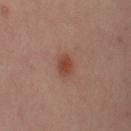Part of a total-body skin-imaging series; this lesion was reviewed on a skin check and was not flagged for biopsy.
Located on the right upper arm.
This image is a 15 mm lesion crop taken from a total-body photograph.
Approximately 2.5 mm at its widest.
A female subject approximately 40 years of age.
This is a cross-polarized tile.
The lesion-visualizer software estimated an eccentricity of roughly 0.65 and a shape-asymmetry score of about 0.2 (0 = symmetric).